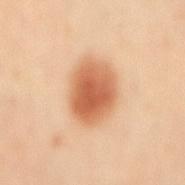<tbp_lesion>
<biopsy_status>not biopsied; imaged during a skin examination</biopsy_status>
<site>back</site>
<patient>
  <sex>male</sex>
  <age_approx>65</age_approx>
</patient>
<lesion_size>
  <long_diameter_mm_approx>6.0</long_diameter_mm_approx>
</lesion_size>
<lighting>cross-polarized</lighting>
<image>
  <source>total-body photography crop</source>
  <field_of_view_mm>15</field_of_view_mm>
</image>
</tbp_lesion>